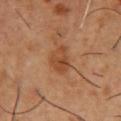Imaged during a routine full-body skin examination; the lesion was not biopsied and no histopathology is available. Longest diameter approximately 3.5 mm. Cropped from a whole-body photographic skin survey; the tile spans about 15 mm. A male subject, in their mid-50s. Located on the chest. Imaged with cross-polarized lighting.